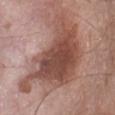Q: Was this lesion biopsied?
A: no biopsy performed (imaged during a skin exam)
Q: What kind of image is this?
A: ~15 mm crop, total-body skin-cancer survey
Q: Where on the body is the lesion?
A: the abdomen
Q: What are the patient's age and sex?
A: male, aged 68–72
Q: What is the lesion's diameter?
A: ~11.5 mm (longest diameter)
Q: How was the tile lit?
A: white-light illumination